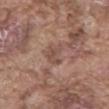The lesion was photographed on a routine skin check and not biopsied; there is no pathology result. A male patient in their mid-70s. A region of skin cropped from a whole-body photographic capture, roughly 15 mm wide. From the front of the torso.